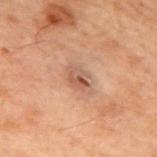This lesion was catalogued during total-body skin photography and was not selected for biopsy. Captured under cross-polarized illumination. A male subject aged approximately 70. Cropped from a total-body skin-imaging series; the visible field is about 15 mm. The recorded lesion diameter is about 3 mm. Located on the upper back. Automated image analysis of the tile measured an average lesion color of about L≈42 a*≈17 b*≈24 (CIELAB) and roughly 8 lightness units darker than nearby skin. The software also gave border irregularity of about 2 on a 0–10 scale, a within-lesion color-variation index near 6/10, and a peripheral color-asymmetry measure near 2.